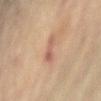  biopsy_status: not biopsied; imaged during a skin examination
  site: left arm
  image:
    source: total-body photography crop
    field_of_view_mm: 15
  lighting: cross-polarized
  patient:
    sex: female
    age_approx: 80
  lesion_size:
    long_diameter_mm_approx: 2.5